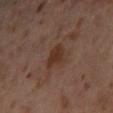| feature | finding |
|---|---|
| workup | no biopsy performed (imaged during a skin exam) |
| tile lighting | cross-polarized illumination |
| acquisition | total-body-photography crop, ~15 mm field of view |
| anatomic site | the left thigh |
| patient | female, about 55 years old |